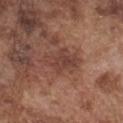{"biopsy_status": "not biopsied; imaged during a skin examination", "lighting": "white-light", "site": "chest", "image": {"source": "total-body photography crop", "field_of_view_mm": 15}, "patient": {"sex": "male", "age_approx": 75}, "lesion_size": {"long_diameter_mm_approx": 4.0}, "automated_metrics": {"border_irregularity_0_10": 3.5, "color_variation_0_10": 3.0, "peripheral_color_asymmetry": 1.0, "nevus_likeness_0_100": 5, "lesion_detection_confidence_0_100": 100}}